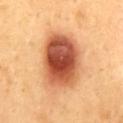notes = catalogued during a skin exam; not biopsied
patient = male, about 50 years old
image source = 15 mm crop, total-body photography
location = the mid back
tile lighting = cross-polarized illumination
TBP lesion metrics = a normalized lesion–skin contrast near 12.5; border irregularity of about 1.5 on a 0–10 scale, a within-lesion color-variation index near 9/10, and radial color variation of about 2.5
size = about 7 mm